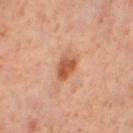No biopsy was performed on this lesion — it was imaged during a full skin examination and was not determined to be concerning. This is a cross-polarized tile. A 15 mm close-up tile from a total-body photography series done for melanoma screening. Located on the right thigh. The subject is a female in their 40s. About 3.5 mm across.